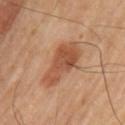Captured during whole-body skin photography for melanoma surveillance; the lesion was not biopsied. Located on the left upper arm. This image is a 15 mm lesion crop taken from a total-body photograph. A male patient in their mid-50s.